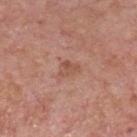Recorded during total-body skin imaging; not selected for excision or biopsy. The lesion is on the chest. This is a white-light tile. Automated image analysis of the tile measured a shape eccentricity near 0.7. The software also gave a border-irregularity index near 3.5/10, a color-variation rating of about 2.5/10, and radial color variation of about 1. A male patient, aged 73 to 77. A close-up tile cropped from a whole-body skin photograph, about 15 mm across.